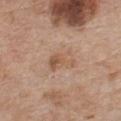biopsy status = catalogued during a skin exam; not biopsied.